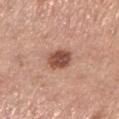<case>
  <biopsy_status>not biopsied; imaged during a skin examination</biopsy_status>
  <patient>
    <sex>female</sex>
    <age_approx>65</age_approx>
  </patient>
  <site>left lower leg</site>
  <image>
    <source>total-body photography crop</source>
    <field_of_view_mm>15</field_of_view_mm>
  </image>
</case>